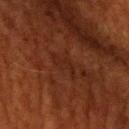Part of a total-body skin-imaging series; this lesion was reviewed on a skin check and was not flagged for biopsy. This image is a 15 mm lesion crop taken from a total-body photograph. On the left upper arm. A male patient, aged approximately 60. The total-body-photography lesion software estimated a normalized lesion–skin contrast near 4.5. It also reported a classifier nevus-likeness of about 0/100 and lesion-presence confidence of about 80/100.